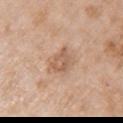workup: catalogued during a skin exam; not biopsied
site: the arm
image source: ~15 mm tile from a whole-body skin photo
size: ~3.5 mm (longest diameter)
subject: female, approximately 70 years of age
automated metrics: an outline eccentricity of about 0.8 (0 = round, 1 = elongated) and a symmetry-axis asymmetry near 0.35; an average lesion color of about L≈59 a*≈20 b*≈31 (CIELAB), a lesion–skin lightness drop of about 9, and a normalized border contrast of about 6
tile lighting: white-light